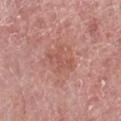A female patient in their 60s. Automated image analysis of the tile measured a lesion area of about 5.5 mm², a shape eccentricity near 0.75, and a shape-asymmetry score of about 0.4 (0 = symmetric). From the arm. Cropped from a whole-body photographic skin survey; the tile spans about 15 mm. Captured under white-light illumination. Longest diameter approximately 3.5 mm.